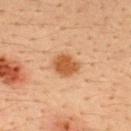notes: imaged on a skin check; not biopsied
lesion diameter: about 3 mm
automated metrics: a lesion area of about 7 mm²; a lesion color around L≈52 a*≈24 b*≈38 in CIELAB, a lesion–skin lightness drop of about 12, and a lesion-to-skin contrast of about 9 (normalized; higher = more distinct); an automated nevus-likeness rating near 100 out of 100
subject: male, about 35 years old
site: the upper back
tile lighting: cross-polarized illumination
image source: ~15 mm crop, total-body skin-cancer survey